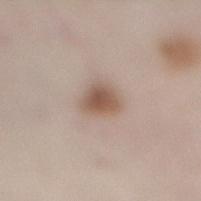follow-up = imaged on a skin check; not biopsied | automated lesion analysis = an average lesion color of about L≈55 a*≈16 b*≈27 (CIELAB) and about 12 CIELAB-L* units darker than the surrounding skin; a classifier nevus-likeness of about 100/100 | lighting = white-light | lesion size = ~3.5 mm (longest diameter) | patient = female, in their 60s | image = ~15 mm tile from a whole-body skin photo | body site = the right lower leg.